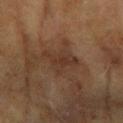Imaged during a routine full-body skin examination; the lesion was not biopsied and no histopathology is available.
The tile uses cross-polarized illumination.
The patient is a female in their 60s.
A roughly 15 mm field-of-view crop from a total-body skin photograph.
Longest diameter approximately 4 mm.
The lesion is on the right forearm.